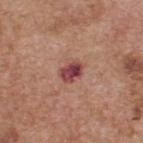{"biopsy_status": "not biopsied; imaged during a skin examination", "patient": {"sex": "male", "age_approx": 60}, "site": "upper back", "image": {"source": "total-body photography crop", "field_of_view_mm": 15}, "automated_metrics": {"area_mm2_approx": 5.0, "shape_asymmetry": 0.2, "cielab_L": 44, "cielab_a": 29, "cielab_b": 22, "vs_skin_darker_L": 14.0, "vs_skin_contrast_norm": 11.0, "nevus_likeness_0_100": 0, "lesion_detection_confidence_0_100": 100}, "lighting": "white-light"}